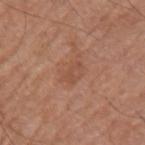workup=no biopsy performed (imaged during a skin exam) | patient=male, roughly 65 years of age | image-analysis metrics=an area of roughly 3 mm², an eccentricity of roughly 0.85, and two-axis asymmetry of about 0.55 | imaging modality=15 mm crop, total-body photography | site=the left upper arm | illumination=white-light illumination | diameter=about 2.5 mm.